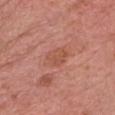Clinical impression:
The lesion was tiled from a total-body skin photograph and was not biopsied.
Context:
From the front of the torso. A close-up tile cropped from a whole-body skin photograph, about 15 mm across. Measured at roughly 3 mm in maximum diameter. The subject is a female roughly 60 years of age. The tile uses white-light illumination.